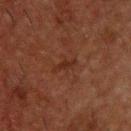– workup — no biopsy performed (imaged during a skin exam)
– body site — the upper back
– illumination — cross-polarized
– patient — male, aged approximately 50
– image source — ~15 mm crop, total-body skin-cancer survey
– automated metrics — a border-irregularity index near 4/10, a color-variation rating of about 0/10, and peripheral color asymmetry of about 0; a nevus-likeness score of about 0/100 and a lesion-detection confidence of about 100/100